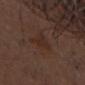Imaged during a routine full-body skin examination; the lesion was not biopsied and no histopathology is available. Captured under white-light illumination. A roughly 15 mm field-of-view crop from a total-body skin photograph. A male patient approximately 75 years of age. Located on the head or neck. About 2.5 mm across.